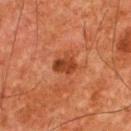The lesion was photographed on a routine skin check and not biopsied; there is no pathology result.
The lesion is located on the back.
This is a cross-polarized tile.
This image is a 15 mm lesion crop taken from a total-body photograph.
Automated image analysis of the tile measured a border-irregularity rating of about 2.5/10 and radial color variation of about 1. It also reported a nevus-likeness score of about 15/100.
The recorded lesion diameter is about 3 mm.
The patient is a male in their mid- to late 60s.